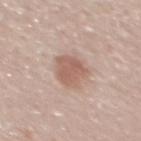A close-up tile cropped from a whole-body skin photograph, about 15 mm across.
Captured under white-light illumination.
Automated tile analysis of the lesion measured an area of roughly 9 mm², a shape eccentricity near 0.6, and a symmetry-axis asymmetry near 0.25. And it measured a within-lesion color-variation index near 2/10 and peripheral color asymmetry of about 0.5. And it measured a nevus-likeness score of about 90/100.
The lesion is located on the upper back.
A male patient, approximately 60 years of age.
Approximately 4 mm at its widest.